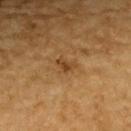Q: Is there a histopathology result?
A: total-body-photography surveillance lesion; no biopsy
Q: What is the anatomic site?
A: the upper back
Q: How was the tile lit?
A: cross-polarized
Q: Automated lesion metrics?
A: a footprint of about 3 mm²; roughly 7 lightness units darker than nearby skin; a border-irregularity index near 4/10 and a peripheral color-asymmetry measure near 0.5; a lesion-detection confidence of about 100/100
Q: What are the patient's age and sex?
A: male, approximately 85 years of age or older
Q: What is the imaging modality?
A: ~15 mm crop, total-body skin-cancer survey
Q: What is the lesion's diameter?
A: ~2.5 mm (longest diameter)